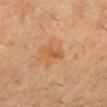This lesion was catalogued during total-body skin photography and was not selected for biopsy. The patient is a female in their 30s. A region of skin cropped from a whole-body photographic capture, roughly 15 mm wide. The lesion is located on the left lower leg.